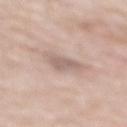Recorded during total-body skin imaging; not selected for excision or biopsy.
The tile uses white-light illumination.
The total-body-photography lesion software estimated an average lesion color of about L≈61 a*≈15 b*≈22 (CIELAB), a lesion–skin lightness drop of about 9, and a normalized lesion–skin contrast near 6. The analysis additionally found an automated nevus-likeness rating near 0 out of 100 and lesion-presence confidence of about 80/100.
A male patient aged 78 to 82.
A region of skin cropped from a whole-body photographic capture, roughly 15 mm wide.
Located on the mid back.